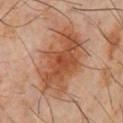About 9 mm across. The patient is a male aged 58–62. Cropped from a total-body skin-imaging series; the visible field is about 15 mm. This is a cross-polarized tile. On the chest.